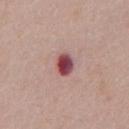biopsy_status: not biopsied; imaged during a skin examination
patient:
  sex: male
  age_approx: 40
site: chest
image:
  source: total-body photography crop
  field_of_view_mm: 15
lesion_size:
  long_diameter_mm_approx: 3.0
lighting: white-light